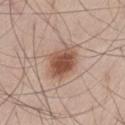The tile uses white-light illumination. Longest diameter approximately 4 mm. A region of skin cropped from a whole-body photographic capture, roughly 15 mm wide. On the leg. The subject is a male aged approximately 50.